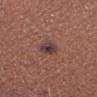Q: Is there a histopathology result?
A: total-body-photography surveillance lesion; no biopsy
Q: Lesion location?
A: the right forearm
Q: How was this image acquired?
A: ~15 mm tile from a whole-body skin photo
Q: Patient demographics?
A: male, approximately 45 years of age